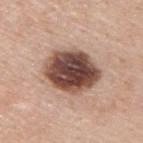follow-up: catalogued during a skin exam; not biopsied
TBP lesion metrics: a lesion area of about 22 mm², an eccentricity of roughly 0.65, and two-axis asymmetry of about 0.15; a mean CIELAB color near L≈46 a*≈20 b*≈24, a lesion–skin lightness drop of about 22, and a lesion-to-skin contrast of about 14.5 (normalized; higher = more distinct); a border-irregularity rating of about 1.5/10, a within-lesion color-variation index near 7.5/10, and radial color variation of about 2
body site: the upper back
subject: male, aged 43 to 47
lighting: white-light illumination
image source: 15 mm crop, total-body photography
lesion diameter: ≈6 mm